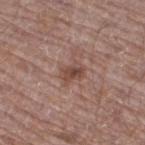<lesion>
<biopsy_status>not biopsied; imaged during a skin examination</biopsy_status>
<automated_metrics>
  <border_irregularity_0_10>1.5</border_irregularity_0_10>
  <color_variation_0_10>4.0</color_variation_0_10>
  <peripheral_color_asymmetry>1.5</peripheral_color_asymmetry>
  <lesion_detection_confidence_0_100>100</lesion_detection_confidence_0_100>
</automated_metrics>
<lighting>white-light</lighting>
<site>right thigh</site>
<patient>
  <sex>male</sex>
  <age_approx>70</age_approx>
</patient>
<lesion_size>
  <long_diameter_mm_approx>2.5</long_diameter_mm_approx>
</lesion_size>
<image>
  <source>total-body photography crop</source>
  <field_of_view_mm>15</field_of_view_mm>
</image>
</lesion>